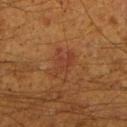<lesion>
<biopsy_status>not biopsied; imaged during a skin examination</biopsy_status>
<image>
  <source>total-body photography crop</source>
  <field_of_view_mm>15</field_of_view_mm>
</image>
<patient>
  <sex>male</sex>
  <age_approx>60</age_approx>
</patient>
<automated_metrics>
  <cielab_L>32</cielab_L>
  <cielab_a>22</cielab_a>
  <cielab_b>28</cielab_b>
  <vs_skin_darker_L>5.0</vs_skin_darker_L>
</automated_metrics>
<site>leg</site>
<lesion_size>
  <long_diameter_mm_approx>3.5</long_diameter_mm_approx>
</lesion_size>
<lighting>cross-polarized</lighting>
</lesion>